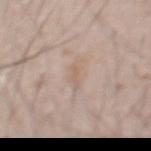A region of skin cropped from a whole-body photographic capture, roughly 15 mm wide.
This is a white-light tile.
The total-body-photography lesion software estimated a lesion color around L≈61 a*≈15 b*≈26 in CIELAB and roughly 6 lightness units darker than nearby skin. The software also gave a border-irregularity index near 5.5/10 and a peripheral color-asymmetry measure near 0.
The lesion is on the front of the torso.
The lesion's longest dimension is about 3 mm.
A male patient, aged approximately 55.